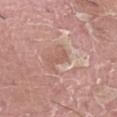Q: What is the anatomic site?
A: the left thigh
Q: What are the patient's age and sex?
A: male, aged 38–42
Q: How was this image acquired?
A: ~15 mm crop, total-body skin-cancer survey